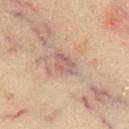The lesion-visualizer software estimated an outline eccentricity of about 0.75 (0 = round, 1 = elongated) and a shape-asymmetry score of about 0.25 (0 = symmetric). This image is a 15 mm lesion crop taken from a total-body photograph. The recorded lesion diameter is about 3.5 mm. From the chest. The patient is a male approximately 65 years of age. Imaged with cross-polarized lighting.